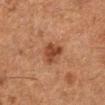workup — imaged on a skin check; not biopsied.